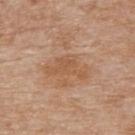Q: Was a biopsy performed?
A: total-body-photography surveillance lesion; no biopsy
Q: How was this image acquired?
A: ~15 mm crop, total-body skin-cancer survey
Q: Lesion location?
A: the upper back
Q: How was the tile lit?
A: white-light
Q: What are the patient's age and sex?
A: female, approximately 75 years of age
Q: Lesion size?
A: ≈5 mm